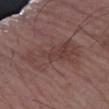Findings:
* follow-up: total-body-photography surveillance lesion; no biopsy
* patient: male, in their mid-60s
* acquisition: total-body-photography crop, ~15 mm field of view
* site: the right forearm
* tile lighting: white-light